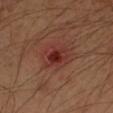| field | value |
|---|---|
| image source | 15 mm crop, total-body photography |
| tile lighting | cross-polarized illumination |
| subject | male, aged around 40 |
| anatomic site | the left upper arm |
| automated lesion analysis | a lesion area of about 11 mm², an eccentricity of roughly 0.6, and two-axis asymmetry of about 0.15; a mean CIELAB color near L≈34 a*≈26 b*≈25, about 8 CIELAB-L* units darker than the surrounding skin, and a normalized lesion–skin contrast near 7; a border-irregularity rating of about 2/10, internal color variation of about 6.5 on a 0–10 scale, and radial color variation of about 1.5; an automated nevus-likeness rating near 0 out of 100 and a lesion-detection confidence of about 100/100 |